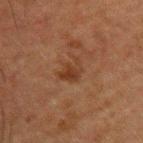notes=no biopsy performed (imaged during a skin exam)
automated lesion analysis=a footprint of about 5 mm² and an eccentricity of roughly 0.7; an average lesion color of about L≈29 a*≈18 b*≈26 (CIELAB) and about 6 CIELAB-L* units darker than the surrounding skin; border irregularity of about 4.5 on a 0–10 scale; an automated nevus-likeness rating near 10 out of 100 and lesion-presence confidence of about 100/100
subject=male, approximately 50 years of age
image=~15 mm crop, total-body skin-cancer survey
anatomic site=the upper back
illumination=cross-polarized